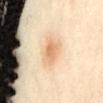This lesion was catalogued during total-body skin photography and was not selected for biopsy. From the back. Cropped from a whole-body photographic skin survey; the tile spans about 15 mm. Approximately 4.5 mm at its widest. An algorithmic analysis of the crop reported a lesion color around L≈75 a*≈20 b*≈39 in CIELAB and a normalized border contrast of about 7. The analysis additionally found a nevus-likeness score of about 100/100 and a lesion-detection confidence of about 100/100. A female subject, aged 38 to 42. The tile uses cross-polarized illumination.